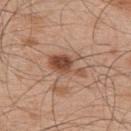{
  "biopsy_status": "not biopsied; imaged during a skin examination",
  "image": {
    "source": "total-body photography crop",
    "field_of_view_mm": 15
  },
  "lighting": "white-light",
  "automated_metrics": {
    "eccentricity": 0.8,
    "shape_asymmetry": 0.4,
    "cielab_L": 50,
    "cielab_a": 21,
    "cielab_b": 30,
    "vs_skin_darker_L": 12.0,
    "nevus_likeness_0_100": 85,
    "lesion_detection_confidence_0_100": 100
  },
  "site": "upper back",
  "patient": {
    "sex": "male",
    "age_approx": 50
  },
  "lesion_size": {
    "long_diameter_mm_approx": 4.5
  }
}